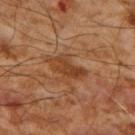Q: Was this lesion biopsied?
A: no biopsy performed (imaged during a skin exam)
Q: What are the patient's age and sex?
A: male, in their mid-50s
Q: How was this image acquired?
A: ~15 mm crop, total-body skin-cancer survey
Q: What did automated image analysis measure?
A: a shape eccentricity near 0.9 and a symmetry-axis asymmetry near 0.3; a classifier nevus-likeness of about 15/100 and a lesion-detection confidence of about 100/100
Q: Illumination type?
A: cross-polarized illumination
Q: What is the lesion's diameter?
A: about 4.5 mm
Q: What is the anatomic site?
A: the right upper arm